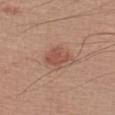Impression:
The lesion was photographed on a routine skin check and not biopsied; there is no pathology result.
Image and clinical context:
About 3.5 mm across. A male subject roughly 60 years of age. This is a white-light tile. The lesion is on the left forearm. The lesion-visualizer software estimated an area of roughly 7 mm², a shape eccentricity near 0.55, and a symmetry-axis asymmetry near 0.3. It also reported a color-variation rating of about 2/10 and radial color variation of about 0.5. A region of skin cropped from a whole-body photographic capture, roughly 15 mm wide.